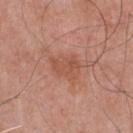{"biopsy_status": "not biopsied; imaged during a skin examination", "site": "front of the torso", "image": {"source": "total-body photography crop", "field_of_view_mm": 15}, "automated_metrics": {"eccentricity": 0.7, "shape_asymmetry": 0.3, "vs_skin_contrast_norm": 5.5, "border_irregularity_0_10": 3.0, "color_variation_0_10": 2.5, "peripheral_color_asymmetry": 1.0}, "patient": {"sex": "male", "age_approx": 55}}